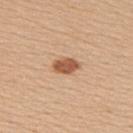biopsy status=catalogued during a skin exam; not biopsied
patient=female, aged around 40
body site=the right upper arm
lighting=white-light illumination
image=total-body-photography crop, ~15 mm field of view
TBP lesion metrics=about 15 CIELAB-L* units darker than the surrounding skin and a normalized border contrast of about 9.5; a border-irregularity rating of about 2/10, a color-variation rating of about 2/10, and a peripheral color-asymmetry measure near 1